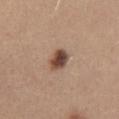{
  "biopsy_status": "not biopsied; imaged during a skin examination",
  "lesion_size": {
    "long_diameter_mm_approx": 3.0
  },
  "image": {
    "source": "total-body photography crop",
    "field_of_view_mm": 15
  },
  "patient": {
    "sex": "female",
    "age_approx": 45
  },
  "site": "front of the torso",
  "lighting": "white-light"
}